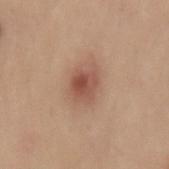Assessment: Imaged during a routine full-body skin examination; the lesion was not biopsied and no histopathology is available. Context: A male subject aged around 30. The lesion-visualizer software estimated a lesion color around L≈53 a*≈22 b*≈28 in CIELAB, roughly 11 lightness units darker than nearby skin, and a lesion-to-skin contrast of about 7.5 (normalized; higher = more distinct). The software also gave a border-irregularity index near 1.5/10, internal color variation of about 5 on a 0–10 scale, and a peripheral color-asymmetry measure near 1.5. It also reported a nevus-likeness score of about 90/100. Approximately 4 mm at its widest. On the mid back. Cropped from a whole-body photographic skin survey; the tile spans about 15 mm. Captured under white-light illumination.